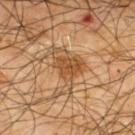Imaged during a routine full-body skin examination; the lesion was not biopsied and no histopathology is available.
A 15 mm close-up extracted from a 3D total-body photography capture.
The lesion is on the upper back.
A male patient, aged 63–67.